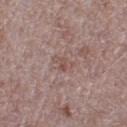  image:
    source: total-body photography crop
    field_of_view_mm: 15
  site: left thigh
  patient:
    sex: male
    age_approx: 70
  lighting: white-light
  lesion_size:
    long_diameter_mm_approx: 2.5